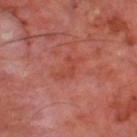No biopsy was performed on this lesion — it was imaged during a full skin examination and was not determined to be concerning. A 15 mm close-up tile from a total-body photography series done for melanoma screening. About 3.5 mm across. The total-body-photography lesion software estimated a lesion area of about 4.5 mm² and a shape-asymmetry score of about 0.5 (0 = symmetric). The analysis additionally found roughly 5 lightness units darker than nearby skin and a normalized border contrast of about 5.5. The software also gave an automated nevus-likeness rating near 0 out of 100 and a lesion-detection confidence of about 100/100. A male patient aged approximately 70. The lesion is located on the left thigh.